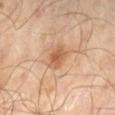Recorded during total-body skin imaging; not selected for excision or biopsy. Cropped from a total-body skin-imaging series; the visible field is about 15 mm. An algorithmic analysis of the crop reported border irregularity of about 2 on a 0–10 scale and a within-lesion color-variation index near 2.5/10. The analysis additionally found a nevus-likeness score of about 55/100 and a lesion-detection confidence of about 100/100. A male subject roughly 65 years of age. Measured at roughly 2.5 mm in maximum diameter. Located on the left lower leg. This is a cross-polarized tile.